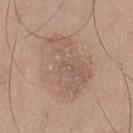follow-up: catalogued during a skin exam; not biopsied | image: total-body-photography crop, ~15 mm field of view | subject: male, aged approximately 60 | anatomic site: the left thigh | image-analysis metrics: an area of roughly 31 mm², a shape eccentricity near 0.7, and a shape-asymmetry score of about 0.25 (0 = symmetric).